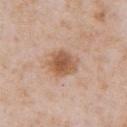Clinical impression:
The lesion was photographed on a routine skin check and not biopsied; there is no pathology result.
Context:
This is a white-light tile. Automated image analysis of the tile measured a lesion color around L≈57 a*≈21 b*≈32 in CIELAB, roughly 12 lightness units darker than nearby skin, and a normalized border contrast of about 8.5. The analysis additionally found a within-lesion color-variation index near 4/10 and a peripheral color-asymmetry measure near 1. Cropped from a total-body skin-imaging series; the visible field is about 15 mm. A male subject aged around 65. About 4 mm across. The lesion is on the abdomen.